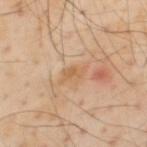Clinical impression: Recorded during total-body skin imaging; not selected for excision or biopsy. Context: The total-body-photography lesion software estimated an average lesion color of about L≈61 a*≈19 b*≈36 (CIELAB) and a normalized lesion–skin contrast near 5.5. It also reported a border-irregularity index near 4/10, a within-lesion color-variation index near 2/10, and a peripheral color-asymmetry measure near 0.5. The software also gave an automated nevus-likeness rating near 0 out of 100 and a lesion-detection confidence of about 100/100. Captured under cross-polarized illumination. From the upper back. A region of skin cropped from a whole-body photographic capture, roughly 15 mm wide. A male subject aged around 55. The lesion's longest dimension is about 3.5 mm.